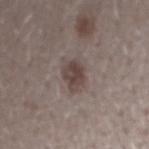Part of a total-body skin-imaging series; this lesion was reviewed on a skin check and was not flagged for biopsy. Approximately 4 mm at its widest. A close-up tile cropped from a whole-body skin photograph, about 15 mm across. On the right forearm. The patient is a male aged 28 to 32. Captured under white-light illumination.